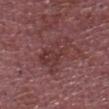biopsy status: imaged on a skin check; not biopsied | subject: male, roughly 65 years of age | image source: 15 mm crop, total-body photography | site: the front of the torso.